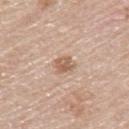Assessment: No biopsy was performed on this lesion — it was imaged during a full skin examination and was not determined to be concerning. Image and clinical context: A lesion tile, about 15 mm wide, cut from a 3D total-body photograph. Imaged with white-light lighting. A male subject aged 58–62. Automated image analysis of the tile measured a border-irregularity index near 2.5/10, internal color variation of about 2.5 on a 0–10 scale, and radial color variation of about 1. From the upper back.